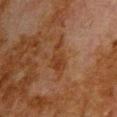No biopsy was performed on this lesion — it was imaged during a full skin examination and was not determined to be concerning.
Longest diameter approximately 4.5 mm.
A male subject aged around 80.
From the upper back.
Imaged with cross-polarized lighting.
Automated image analysis of the tile measured an average lesion color of about L≈30 a*≈19 b*≈28 (CIELAB), about 6 CIELAB-L* units darker than the surrounding skin, and a normalized lesion–skin contrast near 7.
A close-up tile cropped from a whole-body skin photograph, about 15 mm across.